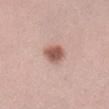Part of a total-body skin-imaging series; this lesion was reviewed on a skin check and was not flagged for biopsy. Approximately 3 mm at its widest. Captured under white-light illumination. A female subject aged 33–37. The lesion-visualizer software estimated a lesion area of about 6 mm², an outline eccentricity of about 0.4 (0 = round, 1 = elongated), and a symmetry-axis asymmetry near 0.15. It also reported a nevus-likeness score of about 100/100. The lesion is on the left forearm. A roughly 15 mm field-of-view crop from a total-body skin photograph.